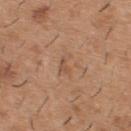Clinical impression:
No biopsy was performed on this lesion — it was imaged during a full skin examination and was not determined to be concerning.
Background:
This image is a 15 mm lesion crop taken from a total-body photograph. Automated image analysis of the tile measured a mean CIELAB color near L≈53 a*≈19 b*≈31 and a lesion–skin lightness drop of about 6. On the upper back. A male patient, in their 40s. About 3 mm across.